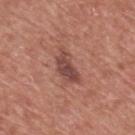| key | value |
|---|---|
| follow-up | imaged on a skin check; not biopsied |
| image-analysis metrics | a within-lesion color-variation index near 4/10 and a peripheral color-asymmetry measure near 1.5; a lesion-detection confidence of about 100/100 |
| location | the mid back |
| illumination | white-light |
| lesion size | ≈4.5 mm |
| subject | male, approximately 75 years of age |
| image | ~15 mm crop, total-body skin-cancer survey |